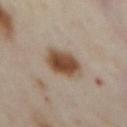The lesion was photographed on a routine skin check and not biopsied; there is no pathology result.
Captured under cross-polarized illumination.
About 4 mm across.
Located on the chest.
The patient is a female approximately 60 years of age.
Cropped from a total-body skin-imaging series; the visible field is about 15 mm.